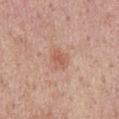{
  "biopsy_status": "not biopsied; imaged during a skin examination",
  "site": "back",
  "lighting": "white-light",
  "automated_metrics": {
    "vs_skin_darker_L": 8.0,
    "vs_skin_contrast_norm": 6.0
  },
  "image": {
    "source": "total-body photography crop",
    "field_of_view_mm": 15
  },
  "patient": {
    "sex": "male",
    "age_approx": 55
  }
}